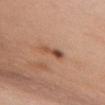workup: total-body-photography surveillance lesion; no biopsy | patient: female, roughly 35 years of age | automated metrics: an area of roughly 4.5 mm² and an outline eccentricity of about 0.95 (0 = round, 1 = elongated); a mean CIELAB color near L≈52 a*≈22 b*≈31 and roughly 11 lightness units darker than nearby skin; a classifier nevus-likeness of about 90/100 and a detector confidence of about 100 out of 100 that the crop contains a lesion | lesion diameter: ≈4 mm | body site: the chest | image source: total-body-photography crop, ~15 mm field of view | lighting: white-light.